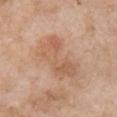Captured during whole-body skin photography for melanoma surveillance; the lesion was not biopsied. A lesion tile, about 15 mm wide, cut from a 3D total-body photograph. The subject is a female aged approximately 75. The tile uses white-light illumination. Located on the right upper arm.